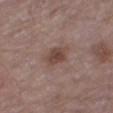workup: no biopsy performed (imaged during a skin exam); patient: male, about 80 years old; image: total-body-photography crop, ~15 mm field of view; anatomic site: the right thigh.